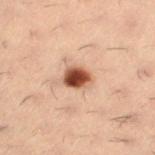No biopsy was performed on this lesion — it was imaged during a full skin examination and was not determined to be concerning. Measured at roughly 3 mm in maximum diameter. The lesion is on the right thigh. Imaged with cross-polarized lighting. An algorithmic analysis of the crop reported a lesion area of about 6 mm², a shape eccentricity near 0.5, and a shape-asymmetry score of about 0.2 (0 = symmetric). The software also gave roughly 18 lightness units darker than nearby skin. And it measured a border-irregularity index near 1.5/10 and a color-variation rating of about 6/10. A male patient, aged around 30. A 15 mm close-up extracted from a 3D total-body photography capture.